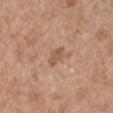Notes:
– workup · total-body-photography surveillance lesion; no biopsy
– lighting · white-light
– subject · female, aged 63–67
– imaging modality · 15 mm crop, total-body photography
– anatomic site · the right upper arm
– automated lesion analysis · a lesion color around L≈55 a*≈20 b*≈31 in CIELAB, about 9 CIELAB-L* units darker than the surrounding skin, and a normalized lesion–skin contrast near 6; a border-irregularity rating of about 3/10
– lesion diameter · about 3 mm